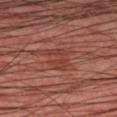Clinical impression: Imaged during a routine full-body skin examination; the lesion was not biopsied and no histopathology is available. Clinical summary: The lesion is on the right lower leg. The tile uses cross-polarized illumination. A male patient aged 43 to 47. This image is a 15 mm lesion crop taken from a total-body photograph.